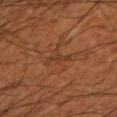This lesion was catalogued during total-body skin photography and was not selected for biopsy.
On the arm.
The subject is a male about 60 years old.
Imaged with cross-polarized lighting.
Cropped from a total-body skin-imaging series; the visible field is about 15 mm.
Approximately 2.5 mm at its widest.